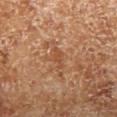Case summary:
• workup · catalogued during a skin exam; not biopsied
• location · the right lower leg
• imaging modality · ~15 mm crop, total-body skin-cancer survey
• lesion size · ~2.5 mm (longest diameter)
• subject · male, approximately 70 years of age